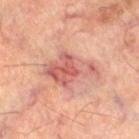Recorded during total-body skin imaging; not selected for excision or biopsy. The recorded lesion diameter is about 6 mm. A 15 mm crop from a total-body photograph taken for skin-cancer surveillance. A male patient, aged approximately 60. Imaged with cross-polarized lighting. On the leg.